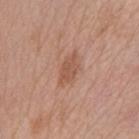acquisition=total-body-photography crop, ~15 mm field of view; tile lighting=white-light; lesion diameter=≈4 mm; site=the chest; subject=female, aged approximately 65.